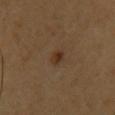<record>
<biopsy_status>not biopsied; imaged during a skin examination</biopsy_status>
<site>upper back</site>
<lighting>cross-polarized</lighting>
<image>
  <source>total-body photography crop</source>
  <field_of_view_mm>15</field_of_view_mm>
</image>
<patient>
  <sex>male</sex>
  <age_approx>65</age_approx>
</patient>
<lesion_size>
  <long_diameter_mm_approx>2.0</long_diameter_mm_approx>
</lesion_size>
<automated_metrics>
  <area_mm2_approx>2.5</area_mm2_approx>
  <eccentricity>0.8</eccentricity>
  <shape_asymmetry>0.35</shape_asymmetry>
  <cielab_L>31</cielab_L>
  <cielab_a>16</cielab_a>
  <cielab_b>28</cielab_b>
  <vs_skin_darker_L>8.0</vs_skin_darker_L>
  <vs_skin_contrast_norm>8.0</vs_skin_contrast_norm>
  <border_irregularity_0_10>3.0</border_irregularity_0_10>
  <color_variation_0_10>1.0</color_variation_0_10>
  <peripheral_color_asymmetry>0.0</peripheral_color_asymmetry>
  <nevus_likeness_0_100>80</nevus_likeness_0_100>
</automated_metrics>
</record>